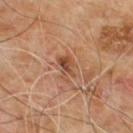Recorded during total-body skin imaging; not selected for excision or biopsy. The lesion is located on the upper back. A close-up tile cropped from a whole-body skin photograph, about 15 mm across. The lesion-visualizer software estimated a shape eccentricity near 0.75. It also reported a normalized border contrast of about 7.5. The analysis additionally found border irregularity of about 4 on a 0–10 scale and a color-variation rating of about 4.5/10. And it measured a classifier nevus-likeness of about 15/100 and lesion-presence confidence of about 100/100. A male subject aged around 60. Imaged with cross-polarized lighting. Longest diameter approximately 2.5 mm.